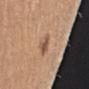Assessment:
Imaged during a routine full-body skin examination; the lesion was not biopsied and no histopathology is available.
Acquisition and patient details:
A male patient, in their mid-60s. Imaged with white-light lighting. On the right upper arm. Cropped from a total-body skin-imaging series; the visible field is about 15 mm. Longest diameter approximately 2.5 mm.